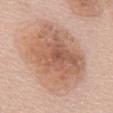No biopsy was performed on this lesion — it was imaged during a full skin examination and was not determined to be concerning. The lesion is on the mid back. This is a white-light tile. A female subject in their 60s. An algorithmic analysis of the crop reported a footprint of about 45 mm², a shape eccentricity near 0.5, and two-axis asymmetry of about 0.15. The analysis additionally found border irregularity of about 2.5 on a 0–10 scale, a color-variation rating of about 5.5/10, and peripheral color asymmetry of about 1.5. The analysis additionally found a classifier nevus-likeness of about 5/100 and a lesion-detection confidence of about 100/100. Longest diameter approximately 8.5 mm. Cropped from a total-body skin-imaging series; the visible field is about 15 mm.